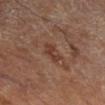The lesion was photographed on a routine skin check and not biopsied; there is no pathology result. Imaged with cross-polarized lighting. The lesion is on the right lower leg. A patient roughly 65 years of age. Cropped from a whole-body photographic skin survey; the tile spans about 15 mm.